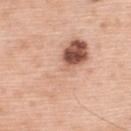biopsy_status: not biopsied; imaged during a skin examination
image:
  source: total-body photography crop
  field_of_view_mm: 15
patient:
  sex: male
  age_approx: 60
lesion_size:
  long_diameter_mm_approx: 9.0
lighting: white-light
site: upper back